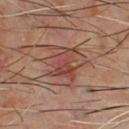This lesion was catalogued during total-body skin photography and was not selected for biopsy.
The recorded lesion diameter is about 4 mm.
Located on the chest.
A 15 mm close-up extracted from a 3D total-body photography capture.
This is a cross-polarized tile.
A male patient, in their 60s.